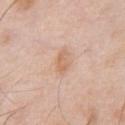Impression:
This lesion was catalogued during total-body skin photography and was not selected for biopsy.